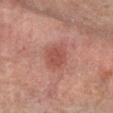Q: Is there a histopathology result?
A: total-body-photography surveillance lesion; no biopsy